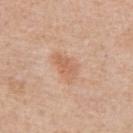Impression:
The lesion was tiled from a total-body skin photograph and was not biopsied.
Image and clinical context:
Automated image analysis of the tile measured a lesion color around L≈62 a*≈22 b*≈33 in CIELAB, roughly 8 lightness units darker than nearby skin, and a normalized lesion–skin contrast near 5.5. And it measured a border-irregularity rating of about 4/10, internal color variation of about 2.5 on a 0–10 scale, and a peripheral color-asymmetry measure near 1. The tile uses white-light illumination. A male subject aged approximately 60. The lesion is located on the right upper arm. A 15 mm crop from a total-body photograph taken for skin-cancer surveillance. Measured at roughly 4 mm in maximum diameter.